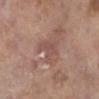Impression: Captured during whole-body skin photography for melanoma surveillance; the lesion was not biopsied. Acquisition and patient details: A female subject aged 83 or over. Cropped from a whole-body photographic skin survey; the tile spans about 15 mm. The lesion is on the right lower leg. Automated tile analysis of the lesion measured an area of roughly 6.5 mm², a shape eccentricity near 0.7, and two-axis asymmetry of about 0.55. And it measured a mean CIELAB color near L≈51 a*≈20 b*≈24, roughly 7 lightness units darker than nearby skin, and a normalized lesion–skin contrast near 5. It also reported a classifier nevus-likeness of about 0/100 and a detector confidence of about 85 out of 100 that the crop contains a lesion. About 3.5 mm across.